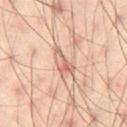Notes:
• workup: imaged on a skin check; not biopsied
• anatomic site: the right thigh
• tile lighting: cross-polarized illumination
• acquisition: total-body-photography crop, ~15 mm field of view
• patient: male, roughly 40 years of age
• lesion size: about 4 mm